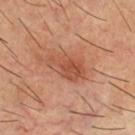The lesion was tiled from a total-body skin photograph and was not biopsied. From the chest. The patient is a male aged around 60. A 15 mm close-up tile from a total-body photography series done for melanoma screening. This is a cross-polarized tile. Measured at roughly 5 mm in maximum diameter.